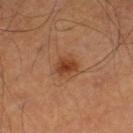This lesion was catalogued during total-body skin photography and was not selected for biopsy.